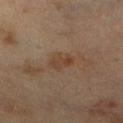Assessment:
Captured during whole-body skin photography for melanoma surveillance; the lesion was not biopsied.
Background:
A female subject, roughly 55 years of age. On the leg. A 15 mm close-up tile from a total-body photography series done for melanoma screening. Automated tile analysis of the lesion measured a mean CIELAB color near L≈35 a*≈15 b*≈25 and a normalized lesion–skin contrast near 6.